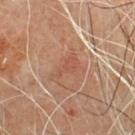Recorded during total-body skin imaging; not selected for excision or biopsy. On the upper back. The tile uses cross-polarized illumination. Approximately 3.5 mm at its widest. A male patient in their 60s. A 15 mm close-up extracted from a 3D total-body photography capture.